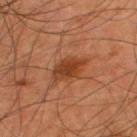Q: Was a biopsy performed?
A: no biopsy performed (imaged during a skin exam)
Q: Who is the patient?
A: male, aged 43 to 47
Q: What did automated image analysis measure?
A: an area of roughly 8 mm², an eccentricity of roughly 0.8, and two-axis asymmetry of about 0.15; an average lesion color of about L≈39 a*≈25 b*≈35 (CIELAB), a lesion–skin lightness drop of about 10, and a lesion-to-skin contrast of about 8.5 (normalized; higher = more distinct); a border-irregularity index near 1.5/10, internal color variation of about 3.5 on a 0–10 scale, and radial color variation of about 1
Q: What kind of image is this?
A: total-body-photography crop, ~15 mm field of view
Q: What is the anatomic site?
A: the upper back